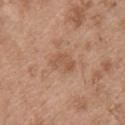Q: Is there a histopathology result?
A: imaged on a skin check; not biopsied
Q: What kind of image is this?
A: ~15 mm tile from a whole-body skin photo
Q: What did automated image analysis measure?
A: two-axis asymmetry of about 0.25; a lesion color around L≈55 a*≈20 b*≈32 in CIELAB, about 7 CIELAB-L* units darker than the surrounding skin, and a normalized border contrast of about 5; internal color variation of about 2.5 on a 0–10 scale and radial color variation of about 1
Q: Patient demographics?
A: male, aged 48 to 52
Q: How was the tile lit?
A: white-light
Q: Where on the body is the lesion?
A: the back